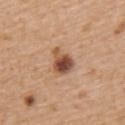The lesion was tiled from a total-body skin photograph and was not biopsied.
Cropped from a total-body skin-imaging series; the visible field is about 15 mm.
On the upper back.
A male patient, aged 68–72.
The tile uses white-light illumination.